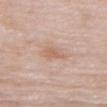Notes:
- notes: no biopsy performed (imaged during a skin exam)
- lighting: white-light
- body site: the left upper arm
- imaging modality: ~15 mm crop, total-body skin-cancer survey
- subject: female, aged around 65
- lesion diameter: about 3 mm